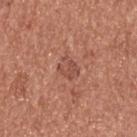Measured at roughly 2.5 mm in maximum diameter. From the upper back. A region of skin cropped from a whole-body photographic capture, roughly 15 mm wide. An algorithmic analysis of the crop reported a lesion–skin lightness drop of about 7 and a lesion-to-skin contrast of about 5.5 (normalized; higher = more distinct). It also reported a border-irregularity index near 5/10, a within-lesion color-variation index near 1/10, and peripheral color asymmetry of about 0.5. And it measured an automated nevus-likeness rating near 0 out of 100 and lesion-presence confidence of about 100/100. The subject is a male aged approximately 55. Imaged with white-light lighting.